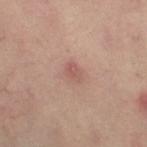Imaged during a routine full-body skin examination; the lesion was not biopsied and no histopathology is available.
A region of skin cropped from a whole-body photographic capture, roughly 15 mm wide.
A female subject aged approximately 40.
The tile uses cross-polarized illumination.
From the right thigh.
Measured at roughly 2.5 mm in maximum diameter.